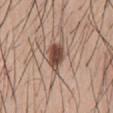workup = no biopsy performed (imaged during a skin exam) | patient = male, in their 30s | image source = ~15 mm tile from a whole-body skin photo | lighting = white-light illumination | body site = the abdomen.